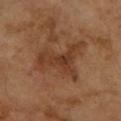No biopsy was performed on this lesion — it was imaged during a full skin examination and was not determined to be concerning.
From the left forearm.
Cropped from a total-body skin-imaging series; the visible field is about 15 mm.
A female patient, approximately 70 years of age.